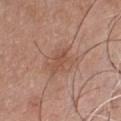workup: no biopsy performed (imaged during a skin exam) | anatomic site: the front of the torso | acquisition: 15 mm crop, total-body photography | automated lesion analysis: a footprint of about 3 mm² and a shape eccentricity near 0.9; a mean CIELAB color near L≈50 a*≈21 b*≈29, a lesion–skin lightness drop of about 7, and a normalized border contrast of about 5.5 | lesion diameter: ≈3 mm | patient: male, aged 43 to 47.